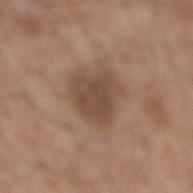follow-up — imaged on a skin check; not biopsied
subject — male, aged approximately 60
imaging modality — ~15 mm tile from a whole-body skin photo
location — the mid back